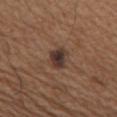Impression: The lesion was photographed on a routine skin check and not biopsied; there is no pathology result. Background: A close-up tile cropped from a whole-body skin photograph, about 15 mm across. The patient is a male roughly 65 years of age. Located on the arm.